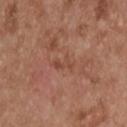Clinical impression: Part of a total-body skin-imaging series; this lesion was reviewed on a skin check and was not flagged for biopsy. Acquisition and patient details: Captured under white-light illumination. Longest diameter approximately 3 mm. The patient is a male about 55 years old. A 15 mm close-up tile from a total-body photography series done for melanoma screening. On the back.